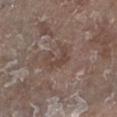<record>
  <image>
    <source>total-body photography crop</source>
    <field_of_view_mm>15</field_of_view_mm>
  </image>
  <patient>
    <sex>male</sex>
    <age_approx>65</age_approx>
  </patient>
  <site>left lower leg</site>
</record>